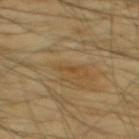biopsy status: catalogued during a skin exam; not biopsied | site: the mid back | image: ~15 mm crop, total-body skin-cancer survey | patient: male, in their mid- to late 60s.